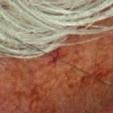Clinical impression:
The lesion was photographed on a routine skin check and not biopsied; there is no pathology result.
Context:
Cropped from a whole-body photographic skin survey; the tile spans about 15 mm. Located on the head or neck. The tile uses cross-polarized illumination. Longest diameter approximately 2.5 mm. A male patient in their 80s.